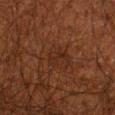notes — no biopsy performed (imaged during a skin exam); image — ~15 mm tile from a whole-body skin photo; TBP lesion metrics — a lesion area of about 4 mm², a shape eccentricity near 0.8, and a shape-asymmetry score of about 0.45 (0 = symmetric); site — the right upper arm; patient — male, aged approximately 50; lesion diameter — ~2.5 mm (longest diameter); lighting — cross-polarized illumination.